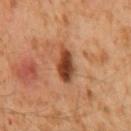Findings:
– biopsy status — total-body-photography surveillance lesion; no biopsy
– automated metrics — a mean CIELAB color near L≈40 a*≈24 b*≈32 and about 15 CIELAB-L* units darker than the surrounding skin; a border-irregularity rating of about 2.5/10 and a color-variation rating of about 6/10; a classifier nevus-likeness of about 90/100
– tile lighting — cross-polarized
– imaging modality — total-body-photography crop, ~15 mm field of view
– site — the mid back
– subject — male, in their 60s